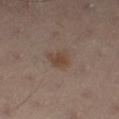Imaged during a routine full-body skin examination; the lesion was not biopsied and no histopathology is available.